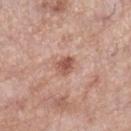Notes:
* follow-up — imaged on a skin check; not biopsied
* patient — female, about 70 years old
* body site — the right lower leg
* image source — total-body-photography crop, ~15 mm field of view